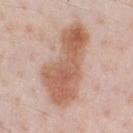Recorded during total-body skin imaging; not selected for excision or biopsy. This image is a 15 mm lesion crop taken from a total-body photograph. Located on the chest. Longest diameter approximately 10 mm. The patient is a male aged around 60.